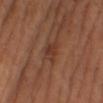biopsy status — imaged on a skin check; not biopsied | subject — female, aged 53 to 57 | imaging modality — ~15 mm tile from a whole-body skin photo | automated lesion analysis — a shape eccentricity near 0.7 and a shape-asymmetry score of about 0.25 (0 = symmetric); a nevus-likeness score of about 0/100 | anatomic site — the right lower leg | tile lighting — cross-polarized illumination | size — about 3 mm.